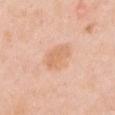Assessment: This lesion was catalogued during total-body skin photography and was not selected for biopsy. Image and clinical context: A region of skin cropped from a whole-body photographic capture, roughly 15 mm wide. The lesion is located on the chest. The recorded lesion diameter is about 4 mm. The patient is a female aged 48 to 52. The total-body-photography lesion software estimated a lesion area of about 7.5 mm², an outline eccentricity of about 0.8 (0 = round, 1 = elongated), and two-axis asymmetry of about 0.25. It also reported a border-irregularity rating of about 2.5/10, a within-lesion color-variation index near 2/10, and peripheral color asymmetry of about 1.